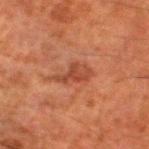Q: Was this lesion biopsied?
A: catalogued during a skin exam; not biopsied
Q: What is the anatomic site?
A: the left lower leg
Q: What did automated image analysis measure?
A: lesion-presence confidence of about 100/100
Q: How was this image acquired?
A: total-body-photography crop, ~15 mm field of view
Q: How large is the lesion?
A: about 4 mm
Q: Patient demographics?
A: male, in their 80s